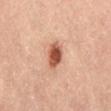Clinical impression: Imaged during a routine full-body skin examination; the lesion was not biopsied and no histopathology is available. Background: Cropped from a total-body skin-imaging series; the visible field is about 15 mm. A female subject, approximately 45 years of age. The lesion's longest dimension is about 3 mm. Automated tile analysis of the lesion measured a lesion area of about 6 mm², a shape eccentricity near 0.65, and a symmetry-axis asymmetry near 0.15. The software also gave an average lesion color of about L≈52 a*≈26 b*≈32 (CIELAB) and a lesion–skin lightness drop of about 17. The analysis additionally found a nevus-likeness score of about 100/100 and a lesion-detection confidence of about 100/100. Imaged with cross-polarized lighting. Located on the lower back.